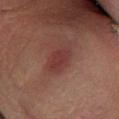Recorded during total-body skin imaging; not selected for excision or biopsy.
Located on the left lower leg.
A male patient, in their mid- to late 60s.
A 15 mm crop from a total-body photograph taken for skin-cancer surveillance.
Automated image analysis of the tile measured a lesion area of about 7.5 mm² and an outline eccentricity of about 0.75 (0 = round, 1 = elongated). It also reported an automated nevus-likeness rating near 45 out of 100 and a lesion-detection confidence of about 100/100.